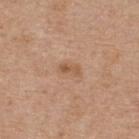biopsy status = no biopsy performed (imaged during a skin exam)
subject = male, about 65 years old
lighting = white-light illumination
site = the upper back
imaging modality = total-body-photography crop, ~15 mm field of view